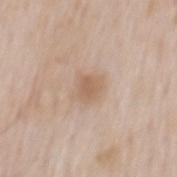<lesion>
  <biopsy_status>not biopsied; imaged during a skin examination</biopsy_status>
  <lighting>white-light</lighting>
  <image>
    <source>total-body photography crop</source>
    <field_of_view_mm>15</field_of_view_mm>
  </image>
  <lesion_size>
    <long_diameter_mm_approx>3.0</long_diameter_mm_approx>
  </lesion_size>
  <patient>
    <sex>male</sex>
    <age_approx>65</age_approx>
  </patient>
  <site>back</site>
  <automated_metrics>
    <area_mm2_approx>6.5</area_mm2_approx>
    <eccentricity>0.5</eccentricity>
    <shape_asymmetry>0.15</shape_asymmetry>
    <border_irregularity_0_10>2.0</border_irregularity_0_10>
    <peripheral_color_asymmetry>0.5</peripheral_color_asymmetry>
    <nevus_likeness_0_100>10</nevus_likeness_0_100>
    <lesion_detection_confidence_0_100>100</lesion_detection_confidence_0_100>
  </automated_metrics>
</lesion>